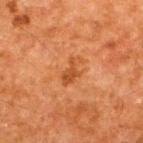Findings:
- notes: catalogued during a skin exam; not biopsied
- automated metrics: a lesion area of about 4.5 mm², an eccentricity of roughly 0.85, and a symmetry-axis asymmetry near 0.4; an average lesion color of about L≈39 a*≈24 b*≈35 (CIELAB); a nevus-likeness score of about 5/100 and a detector confidence of about 100 out of 100 that the crop contains a lesion
- acquisition: ~15 mm crop, total-body skin-cancer survey
- tile lighting: cross-polarized illumination
- anatomic site: the upper back
- patient: male, roughly 60 years of age